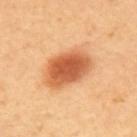The lesion was photographed on a routine skin check and not biopsied; there is no pathology result.
The lesion-visualizer software estimated a lesion–skin lightness drop of about 16. And it measured internal color variation of about 5 on a 0–10 scale and a peripheral color-asymmetry measure near 1.5.
Located on the upper back.
Measured at roughly 6 mm in maximum diameter.
The patient is female.
This image is a 15 mm lesion crop taken from a total-body photograph.
Captured under cross-polarized illumination.